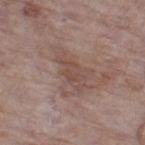Findings:
* follow-up: total-body-photography surveillance lesion; no biopsy
* image-analysis metrics: a mean CIELAB color near L≈48 a*≈17 b*≈23, a lesion–skin lightness drop of about 7, and a normalized border contrast of about 5.5; radial color variation of about 0.5; a lesion-detection confidence of about 85/100
* lesion size: ~4.5 mm (longest diameter)
* body site: the left thigh
* patient: female, aged around 85 or older
* imaging modality: ~15 mm tile from a whole-body skin photo